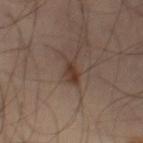Q: Is there a histopathology result?
A: imaged on a skin check; not biopsied
Q: Patient demographics?
A: male, aged 48 to 52
Q: Lesion size?
A: ≈4 mm
Q: What kind of image is this?
A: 15 mm crop, total-body photography
Q: What is the anatomic site?
A: the left leg